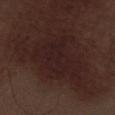An algorithmic analysis of the crop reported an area of roughly 27 mm², an outline eccentricity of about 0.6 (0 = round, 1 = elongated), and a symmetry-axis asymmetry near 0.4. The analysis additionally found an average lesion color of about L≈20 a*≈17 b*≈16 (CIELAB), a lesion–skin lightness drop of about 4, and a normalized lesion–skin contrast near 6.5. It also reported border irregularity of about 7 on a 0–10 scale and peripheral color asymmetry of about 0.5. The software also gave a classifier nevus-likeness of about 0/100 and lesion-presence confidence of about 95/100. A 15 mm close-up extracted from a 3D total-body photography capture. Longest diameter approximately 7.5 mm. Imaged with white-light lighting. On the leg. The subject is a male in their 70s.